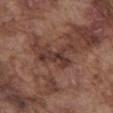{
  "biopsy_status": "not biopsied; imaged during a skin examination",
  "site": "front of the torso",
  "lesion_size": {
    "long_diameter_mm_approx": 5.0
  },
  "lighting": "white-light",
  "patient": {
    "sex": "male",
    "age_approx": 75
  },
  "image": {
    "source": "total-body photography crop",
    "field_of_view_mm": 15
  },
  "automated_metrics": {
    "area_mm2_approx": 8.0,
    "shape_asymmetry": 0.6,
    "cielab_L": 36,
    "cielab_a": 19,
    "cielab_b": 23,
    "vs_skin_darker_L": 9.0,
    "vs_skin_contrast_norm": 8.0,
    "color_variation_0_10": 3.5,
    "peripheral_color_asymmetry": 1.5,
    "nevus_likeness_0_100": 0,
    "lesion_detection_confidence_0_100": 70
  }
}